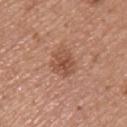The lesion was photographed on a routine skin check and not biopsied; there is no pathology result.
Measured at roughly 3.5 mm in maximum diameter.
A male subject aged 53 to 57.
This image is a 15 mm lesion crop taken from a total-body photograph.
An algorithmic analysis of the crop reported a footprint of about 7 mm², an eccentricity of roughly 0.4, and a symmetry-axis asymmetry near 0.25. The analysis additionally found a lesion color around L≈51 a*≈23 b*≈30 in CIELAB, about 9 CIELAB-L* units darker than the surrounding skin, and a normalized lesion–skin contrast near 6.5. The software also gave a border-irregularity index near 3.5/10 and internal color variation of about 4 on a 0–10 scale. It also reported a nevus-likeness score of about 60/100.
The lesion is on the arm.